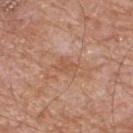follow-up = total-body-photography surveillance lesion; no biopsy | tile lighting = white-light | lesion diameter = about 2.5 mm | patient = male, roughly 80 years of age | imaging modality = 15 mm crop, total-body photography | site = the upper back.